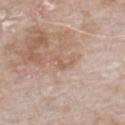This lesion was catalogued during total-body skin photography and was not selected for biopsy.
A male subject aged approximately 80.
Cropped from a whole-body photographic skin survey; the tile spans about 15 mm.
The lesion is on the chest.